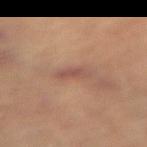Assessment: Captured during whole-body skin photography for melanoma surveillance; the lesion was not biopsied. Acquisition and patient details: A female patient, approximately 65 years of age. The tile uses cross-polarized illumination. On the left leg. A 15 mm crop from a total-body photograph taken for skin-cancer surveillance.